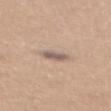Findings:
– biopsy status: imaged on a skin check; not biopsied
– site: the upper back
– diameter: ~4 mm (longest diameter)
– image: 15 mm crop, total-body photography
– patient: female, about 30 years old